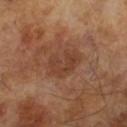<lesion>
<image>
  <source>total-body photography crop</source>
  <field_of_view_mm>15</field_of_view_mm>
</image>
<automated_metrics>
  <cielab_L>39</cielab_L>
  <cielab_a>21</cielab_a>
  <cielab_b>29</cielab_b>
  <vs_skin_darker_L>6.0</vs_skin_darker_L>
  <vs_skin_contrast_norm>6.0</vs_skin_contrast_norm>
  <border_irregularity_0_10>4.5</border_irregularity_0_10>
  <color_variation_0_10>3.0</color_variation_0_10>
  <peripheral_color_asymmetry>1.0</peripheral_color_asymmetry>
  <nevus_likeness_0_100>0</nevus_likeness_0_100>
  <lesion_detection_confidence_0_100>100</lesion_detection_confidence_0_100>
</automated_metrics>
<lesion_size>
  <long_diameter_mm_approx>4.0</long_diameter_mm_approx>
</lesion_size>
<patient>
  <sex>male</sex>
  <age_approx>65</age_approx>
</patient>
<lighting>cross-polarized</lighting>
</lesion>